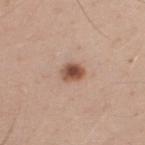| key | value |
|---|---|
| biopsy status | no biopsy performed (imaged during a skin exam) |
| acquisition | ~15 mm tile from a whole-body skin photo |
| location | the upper back |
| tile lighting | white-light |
| size | ≈2.5 mm |
| subject | male, roughly 40 years of age |
| automated lesion analysis | an area of roughly 4.5 mm², a shape eccentricity near 0.6, and two-axis asymmetry of about 0.3; border irregularity of about 2 on a 0–10 scale and a within-lesion color-variation index near 4/10; a lesion-detection confidence of about 100/100 |